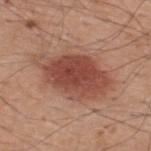- biopsy status · no biopsy performed (imaged during a skin exam)
- image · 15 mm crop, total-body photography
- anatomic site · the back
- subject · male, about 60 years old
- automated lesion analysis · a lesion area of about 27 mm² and two-axis asymmetry of about 0.2; a border-irregularity index near 3/10 and internal color variation of about 4.5 on a 0–10 scale; lesion-presence confidence of about 100/100
- lighting · white-light
- diameter · ~8.5 mm (longest diameter)